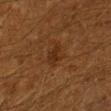The lesion was photographed on a routine skin check and not biopsied; there is no pathology result.
A roughly 15 mm field-of-view crop from a total-body skin photograph.
This is a cross-polarized tile.
The patient is a male about 60 years old.
The total-body-photography lesion software estimated a border-irregularity index near 2.5/10, a within-lesion color-variation index near 2.5/10, and radial color variation of about 1. It also reported a lesion-detection confidence of about 100/100.
Measured at roughly 3 mm in maximum diameter.
The lesion is on the left lower leg.